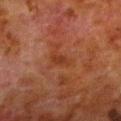workup: catalogued during a skin exam; not biopsied | body site: the left lower leg | subject: male, roughly 80 years of age | image: ~15 mm crop, total-body skin-cancer survey.